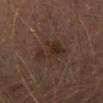follow-up — total-body-photography surveillance lesion; no biopsy | tile lighting — cross-polarized illumination | TBP lesion metrics — roughly 6 lightness units darker than nearby skin and a normalized border contrast of about 7 | lesion diameter — ~4.5 mm (longest diameter) | anatomic site — the right thigh | imaging modality — total-body-photography crop, ~15 mm field of view | subject — male, aged approximately 65.